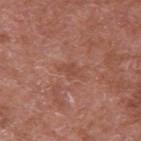workup: imaged on a skin check; not biopsied | automated lesion analysis: an eccentricity of roughly 0.75 and a shape-asymmetry score of about 0.35 (0 = symmetric); a lesion–skin lightness drop of about 6 and a normalized lesion–skin contrast near 4.5 | body site: the right upper arm | size: ≈2.5 mm | subject: male, in their mid-60s | acquisition: ~15 mm tile from a whole-body skin photo.